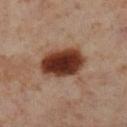biopsy status = no biopsy performed (imaged during a skin exam) | site = the left lower leg | subject = female, roughly 55 years of age | illumination = cross-polarized | image source = 15 mm crop, total-body photography.